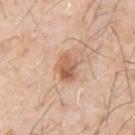Assessment: Part of a total-body skin-imaging series; this lesion was reviewed on a skin check and was not flagged for biopsy.